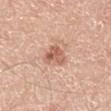Assessment: Part of a total-body skin-imaging series; this lesion was reviewed on a skin check and was not flagged for biopsy. Context: Imaged with white-light lighting. A close-up tile cropped from a whole-body skin photograph, about 15 mm across. Located on the left lower leg. The recorded lesion diameter is about 3.5 mm. A male subject, aged around 70.